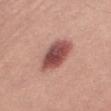workup: no biopsy performed (imaged during a skin exam); patient: female, aged 28–32; imaging modality: total-body-photography crop, ~15 mm field of view; tile lighting: white-light illumination; body site: the leg.